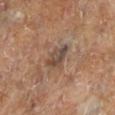| field | value |
|---|---|
| biopsy status | catalogued during a skin exam; not biopsied |
| imaging modality | total-body-photography crop, ~15 mm field of view |
| size | ≈3 mm |
| lighting | cross-polarized |
| subject | female |
| location | the leg |